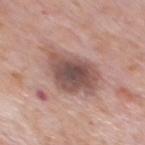patient — male, aged 78 to 82; image source — 15 mm crop, total-body photography; location — the chest.